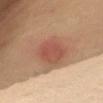Acquisition and patient details:
A close-up tile cropped from a whole-body skin photograph, about 15 mm across. About 7 mm across. Imaged with cross-polarized lighting. A female subject, aged 48 to 52. An algorithmic analysis of the crop reported an area of roughly 24 mm², an eccentricity of roughly 0.75, and a symmetry-axis asymmetry near 0.25. And it measured a lesion color around L≈56 a*≈22 b*≈31 in CIELAB and a lesion–skin lightness drop of about 11. And it measured a lesion-detection confidence of about 100/100. Located on the chest.